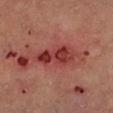subject = male, in their mid- to late 50s | image-analysis metrics = a lesion area of about 14 mm², an eccentricity of roughly 0.9, and two-axis asymmetry of about 0.25; an average lesion color of about L≈34 a*≈28 b*≈23 (CIELAB), a lesion–skin lightness drop of about 10, and a normalized border contrast of about 8.5; border irregularity of about 3 on a 0–10 scale, a within-lesion color-variation index near 6.5/10, and a peripheral color-asymmetry measure near 2.5 | diameter = ~6.5 mm (longest diameter) | image = ~15 mm crop, total-body skin-cancer survey | location = the left lower leg.